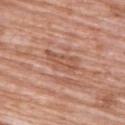Assessment: The lesion was photographed on a routine skin check and not biopsied; there is no pathology result. Acquisition and patient details: A close-up tile cropped from a whole-body skin photograph, about 15 mm across. A female subject, aged approximately 70. The lesion is on the upper back.